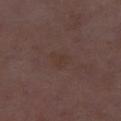| field | value |
|---|---|
| biopsy status | catalogued during a skin exam; not biopsied |
| size | ≈3 mm |
| illumination | white-light illumination |
| site | the leg |
| patient | female, approximately 30 years of age |
| acquisition | 15 mm crop, total-body photography |